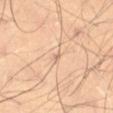Part of a total-body skin-imaging series; this lesion was reviewed on a skin check and was not flagged for biopsy.
About 1.5 mm across.
Imaged with cross-polarized lighting.
A male subject, aged approximately 70.
The lesion is on the left thigh.
A lesion tile, about 15 mm wide, cut from a 3D total-body photograph.
Automated tile analysis of the lesion measured a symmetry-axis asymmetry near 0.35. The software also gave a mean CIELAB color near L≈65 a*≈18 b*≈30, about 9 CIELAB-L* units darker than the surrounding skin, and a lesion-to-skin contrast of about 5.5 (normalized; higher = more distinct). It also reported a classifier nevus-likeness of about 0/100 and a detector confidence of about 30 out of 100 that the crop contains a lesion.